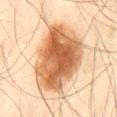The lesion was photographed on a routine skin check and not biopsied; there is no pathology result. Approximately 9 mm at its widest. A male subject, aged approximately 45. Automated tile analysis of the lesion measured a lesion area of about 41 mm², an outline eccentricity of about 0.75 (0 = round, 1 = elongated), and a symmetry-axis asymmetry near 0.2. It also reported border irregularity of about 2.5 on a 0–10 scale and internal color variation of about 7.5 on a 0–10 scale. Captured under cross-polarized illumination. The lesion is located on the abdomen. A close-up tile cropped from a whole-body skin photograph, about 15 mm across.